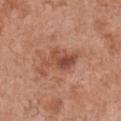Case summary:
* biopsy status · no biopsy performed (imaged during a skin exam)
* image source · 15 mm crop, total-body photography
* body site · the chest
* subject · female, in their mid- to late 60s
* lighting · white-light